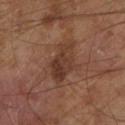Q: Was a biopsy performed?
A: catalogued during a skin exam; not biopsied
Q: Automated lesion metrics?
A: a footprint of about 11 mm², an outline eccentricity of about 0.85 (0 = round, 1 = elongated), and a symmetry-axis asymmetry near 0.25
Q: Lesion size?
A: ≈5 mm
Q: Patient demographics?
A: male, aged approximately 65
Q: What lighting was used for the tile?
A: cross-polarized
Q: What is the imaging modality?
A: total-body-photography crop, ~15 mm field of view